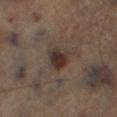Context: The patient is a male aged 63–67. The tile uses cross-polarized illumination. Automated tile analysis of the lesion measured a lesion area of about 6 mm², a shape eccentricity near 0.45, and two-axis asymmetry of about 0.3. The analysis additionally found an average lesion color of about L≈29 a*≈12 b*≈18 (CIELAB), roughly 9 lightness units darker than nearby skin, and a normalized border contrast of about 9.5. And it measured a classifier nevus-likeness of about 90/100 and lesion-presence confidence of about 100/100. A 15 mm close-up tile from a total-body photography series done for melanoma screening. The lesion's longest dimension is about 3 mm. The lesion is on the left lower leg.